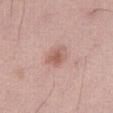Q: How was the tile lit?
A: white-light
Q: What is the imaging modality?
A: ~15 mm crop, total-body skin-cancer survey
Q: Lesion location?
A: the abdomen
Q: What are the patient's age and sex?
A: female, about 50 years old
Q: Automated lesion metrics?
A: an area of roughly 5 mm² and a shape-asymmetry score of about 0.3 (0 = symmetric); a mean CIELAB color near L≈59 a*≈22 b*≈27, a lesion–skin lightness drop of about 9, and a normalized border contrast of about 6.5; a nevus-likeness score of about 40/100